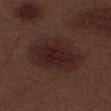* notes · no biopsy performed (imaged during a skin exam)
* image · ~15 mm crop, total-body skin-cancer survey
* anatomic site · the left thigh
* illumination · white-light
* automated metrics · about 6 CIELAB-L* units darker than the surrounding skin and a normalized lesion–skin contrast near 8; a border-irregularity index near 1.5/10, a within-lesion color-variation index near 3/10, and peripheral color asymmetry of about 1
* patient · male, roughly 70 years of age